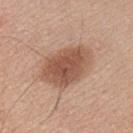– automated metrics: a peripheral color-asymmetry measure near 1
– patient: male, aged approximately 30
– illumination: white-light illumination
– acquisition: ~15 mm crop, total-body skin-cancer survey
– body site: the right upper arm